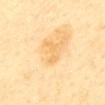Background:
A 15 mm close-up extracted from a 3D total-body photography capture. A female subject aged 58 to 62. On the mid back.